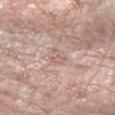Notes:
- location · the left forearm
- acquisition · total-body-photography crop, ~15 mm field of view
- illumination · white-light illumination
- subject · female, aged 68–72